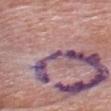Histopathology of the biopsied lesion showed a nodular basal cell carcinoma (malignant).